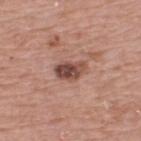The lesion was photographed on a routine skin check and not biopsied; there is no pathology result. Automated image analysis of the tile measured a mean CIELAB color near L≈47 a*≈22 b*≈24, roughly 14 lightness units darker than nearby skin, and a normalized lesion–skin contrast near 10. And it measured an automated nevus-likeness rating near 65 out of 100 and a detector confidence of about 100 out of 100 that the crop contains a lesion. From the upper back. A male patient aged around 75. Cropped from a whole-body photographic skin survey; the tile spans about 15 mm. Measured at roughly 3.5 mm in maximum diameter.